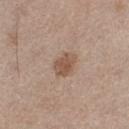Findings:
• follow-up — no biopsy performed (imaged during a skin exam)
• automated lesion analysis — a normalized lesion–skin contrast near 7.5; border irregularity of about 2 on a 0–10 scale, a color-variation rating of about 2.5/10, and a peripheral color-asymmetry measure near 1
• site — the left thigh
• lighting — white-light
• imaging modality — ~15 mm crop, total-body skin-cancer survey
• subject — female, about 50 years old
• size — ≈3 mm